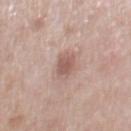Measured at roughly 3 mm in maximum diameter. A male patient about 50 years old. The lesion is located on the chest. The lesion-visualizer software estimated an outline eccentricity of about 0.65 (0 = round, 1 = elongated) and a shape-asymmetry score of about 0.25 (0 = symmetric). A 15 mm crop from a total-body photograph taken for skin-cancer surveillance.